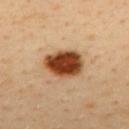Impression:
This lesion was catalogued during total-body skin photography and was not selected for biopsy.
Clinical summary:
The lesion-visualizer software estimated a mean CIELAB color near L≈45 a*≈26 b*≈37, roughly 23 lightness units darker than nearby skin, and a normalized border contrast of about 15. This is a cross-polarized tile. A male subject, aged 38–42. Cropped from a total-body skin-imaging series; the visible field is about 15 mm. On the back.